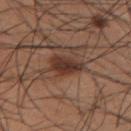Q: Was this lesion biopsied?
A: no biopsy performed (imaged during a skin exam)
Q: How was this image acquired?
A: ~15 mm tile from a whole-body skin photo
Q: Lesion location?
A: the right upper arm
Q: How was the tile lit?
A: white-light illumination
Q: Patient demographics?
A: male, aged around 35
Q: How large is the lesion?
A: ~4.5 mm (longest diameter)